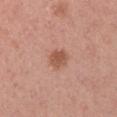Impression: The lesion was tiled from a total-body skin photograph and was not biopsied. Clinical summary: A region of skin cropped from a whole-body photographic capture, roughly 15 mm wide. An algorithmic analysis of the crop reported a within-lesion color-variation index near 2/10 and peripheral color asymmetry of about 0.5. Imaged with white-light lighting. A male subject, approximately 40 years of age. Longest diameter approximately 2.5 mm. Located on the left upper arm.